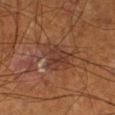Imaged during a routine full-body skin examination; the lesion was not biopsied and no histopathology is available. On the right thigh. Cropped from a whole-body photographic skin survey; the tile spans about 15 mm. Longest diameter approximately 4.5 mm. The patient is a male roughly 70 years of age. Captured under cross-polarized illumination.